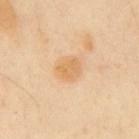| feature | finding |
|---|---|
| notes | catalogued during a skin exam; not biopsied |
| location | the mid back |
| automated lesion analysis | a border-irregularity index near 1.5/10 and a color-variation rating of about 2.5/10 |
| subject | male, aged around 50 |
| lesion diameter | about 2.5 mm |
| image source | 15 mm crop, total-body photography |
| illumination | cross-polarized illumination |